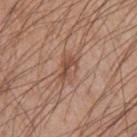The subject is a male aged 48 to 52.
From the arm.
Automated tile analysis of the lesion measured an area of roughly 5.5 mm² and an eccentricity of roughly 0.8. The analysis additionally found a border-irregularity index near 3/10, internal color variation of about 5.5 on a 0–10 scale, and radial color variation of about 2.
This is a white-light tile.
A 15 mm crop from a total-body photograph taken for skin-cancer surveillance.
Longest diameter approximately 3 mm.